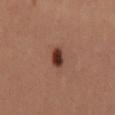| key | value |
|---|---|
| follow-up | imaged on a skin check; not biopsied |
| patient | female, approximately 35 years of age |
| image | ~15 mm crop, total-body skin-cancer survey |
| lesion size | ≈3 mm |
| automated metrics | an average lesion color of about L≈36 a*≈23 b*≈27 (CIELAB), a lesion–skin lightness drop of about 15, and a lesion-to-skin contrast of about 12.5 (normalized; higher = more distinct); an automated nevus-likeness rating near 100 out of 100 and lesion-presence confidence of about 100/100 |
| illumination | cross-polarized illumination |
| location | the right thigh |